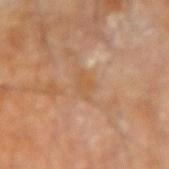Measured at roughly 2.5 mm in maximum diameter. The lesion is located on the left forearm. The total-body-photography lesion software estimated a mean CIELAB color near L≈54 a*≈20 b*≈36, about 5 CIELAB-L* units darker than the surrounding skin, and a normalized lesion–skin contrast near 5.5. It also reported border irregularity of about 3.5 on a 0–10 scale, a color-variation rating of about 1.5/10, and a peripheral color-asymmetry measure near 0.5. It also reported an automated nevus-likeness rating near 0 out of 100. The subject is a male roughly 85 years of age or older. The tile uses cross-polarized illumination. This image is a 15 mm lesion crop taken from a total-body photograph.